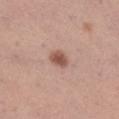Context: The subject is a female aged around 30. Located on the right lower leg. A lesion tile, about 15 mm wide, cut from a 3D total-body photograph.